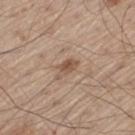• follow-up: catalogued during a skin exam; not biopsied
• subject: male, roughly 60 years of age
• site: the leg
• TBP lesion metrics: a shape-asymmetry score of about 0.4 (0 = symmetric); an average lesion color of about L≈53 a*≈17 b*≈28 (CIELAB), about 10 CIELAB-L* units darker than the surrounding skin, and a normalized lesion–skin contrast near 7
• imaging modality: total-body-photography crop, ~15 mm field of view
• lighting: white-light illumination
• lesion size: ~3.5 mm (longest diameter)